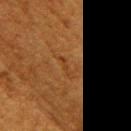Assessment: Captured during whole-body skin photography for melanoma surveillance; the lesion was not biopsied. Clinical summary: About 2.5 mm across. The total-body-photography lesion software estimated about 5 CIELAB-L* units darker than the surrounding skin and a lesion-to-skin contrast of about 5 (normalized; higher = more distinct). The analysis additionally found a nevus-likeness score of about 0/100 and a detector confidence of about 80 out of 100 that the crop contains a lesion. Cropped from a total-body skin-imaging series; the visible field is about 15 mm. On the right upper arm. The patient is a female aged approximately 50.